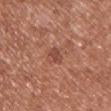follow-up=total-body-photography surveillance lesion; no biopsy | lighting=white-light illumination | site=the chest | subject=female, aged 38–42 | size=~2.5 mm (longest diameter) | image=~15 mm crop, total-body skin-cancer survey.